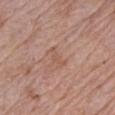{"biopsy_status": "not biopsied; imaged during a skin examination", "automated_metrics": {"area_mm2_approx": 2.5, "shape_asymmetry": 0.6, "border_irregularity_0_10": 6.5, "color_variation_0_10": 0.0, "peripheral_color_asymmetry": 0.0}, "lighting": "white-light", "image": {"source": "total-body photography crop", "field_of_view_mm": 15}, "site": "left upper arm", "patient": {"sex": "male", "age_approx": 70}, "lesion_size": {"long_diameter_mm_approx": 2.5}}